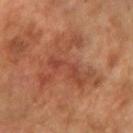Imaged during a routine full-body skin examination; the lesion was not biopsied and no histopathology is available.
This image is a 15 mm lesion crop taken from a total-body photograph.
The patient is a female aged 68 to 72.
Captured under cross-polarized illumination.
An algorithmic analysis of the crop reported an area of roughly 24 mm², an outline eccentricity of about 0.65 (0 = round, 1 = elongated), and a shape-asymmetry score of about 0.7 (0 = symmetric). And it measured a border-irregularity rating of about 10/10, a within-lesion color-variation index near 4.5/10, and a peripheral color-asymmetry measure near 1.5.
Measured at roughly 8 mm in maximum diameter.
On the right forearm.